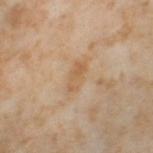Assessment:
The lesion was photographed on a routine skin check and not biopsied; there is no pathology result.
Background:
The total-body-photography lesion software estimated an area of roughly 5 mm² and an eccentricity of roughly 0.9. And it measured an average lesion color of about L≈60 a*≈17 b*≈36 (CIELAB) and a normalized lesion–skin contrast near 5.5. The analysis additionally found a border-irregularity index near 3/10, a color-variation rating of about 3.5/10, and radial color variation of about 1. Longest diameter approximately 3.5 mm. The lesion is located on the left thigh. The subject is a female in their mid- to late 50s. Captured under cross-polarized illumination. A 15 mm crop from a total-body photograph taken for skin-cancer surveillance.